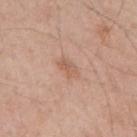This lesion was catalogued during total-body skin photography and was not selected for biopsy.
A close-up tile cropped from a whole-body skin photograph, about 15 mm across.
A male patient, in their 70s.
The tile uses white-light illumination.
The lesion is on the right upper arm.